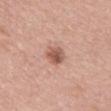{"biopsy_status": "not biopsied; imaged during a skin examination", "image": {"source": "total-body photography crop", "field_of_view_mm": 15}, "automated_metrics": {"area_mm2_approx": 5.0, "eccentricity": 0.55, "shape_asymmetry": 0.2, "nevus_likeness_0_100": 85, "lesion_detection_confidence_0_100": 100}, "patient": {"sex": "male", "age_approx": 65}, "site": "chest"}